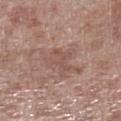Q: Was this lesion biopsied?
A: total-body-photography surveillance lesion; no biopsy
Q: What kind of image is this?
A: ~15 mm crop, total-body skin-cancer survey
Q: What did automated image analysis measure?
A: a lesion area of about 6 mm², an outline eccentricity of about 0.8 (0 = round, 1 = elongated), and two-axis asymmetry of about 0.45; a lesion color around L≈52 a*≈19 b*≈24 in CIELAB, a lesion–skin lightness drop of about 6, and a normalized lesion–skin contrast near 4.5; border irregularity of about 5.5 on a 0–10 scale, a color-variation rating of about 2/10, and a peripheral color-asymmetry measure near 0.5; a nevus-likeness score of about 0/100 and a detector confidence of about 100 out of 100 that the crop contains a lesion
Q: What is the lesion's diameter?
A: ≈4 mm
Q: What lighting was used for the tile?
A: white-light illumination
Q: Patient demographics?
A: male, in their mid- to late 50s
Q: Where on the body is the lesion?
A: the left lower leg